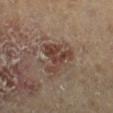Background: The lesion's longest dimension is about 4.5 mm. The subject is a male approximately 85 years of age or older. From the leg. Imaged with cross-polarized lighting. A roughly 15 mm field-of-view crop from a total-body skin photograph.